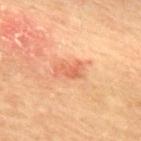Imaged during a routine full-body skin examination; the lesion was not biopsied and no histopathology is available. A region of skin cropped from a whole-body photographic capture, roughly 15 mm wide. The lesion is located on the upper back. This is a cross-polarized tile. The recorded lesion diameter is about 3 mm. The patient is a female aged 78–82.